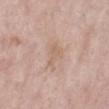biopsy_status: not biopsied; imaged during a skin examination
site: left lower leg
patient:
  sex: female
  age_approx: 70
lighting: white-light
image:
  source: total-body photography crop
  field_of_view_mm: 15
lesion_size:
  long_diameter_mm_approx: 3.0
automated_metrics:
  area_mm2_approx: 5.0
  eccentricity: 0.7
  shape_asymmetry: 0.5
  vs_skin_darker_L: 6.0
  vs_skin_contrast_norm: 4.5
  border_irregularity_0_10: 5.0
  peripheral_color_asymmetry: 0.5
  lesion_detection_confidence_0_100: 100